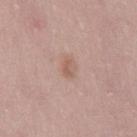Case summary:
• biopsy status — imaged on a skin check; not biopsied
• lesion size — about 2.5 mm
• body site — the leg
• acquisition — ~15 mm tile from a whole-body skin photo
• subject — female, approximately 30 years of age
• lighting — white-light illumination